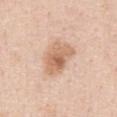<tbp_lesion>
<biopsy_status>not biopsied; imaged during a skin examination</biopsy_status>
<image>
  <source>total-body photography crop</source>
  <field_of_view_mm>15</field_of_view_mm>
</image>
<patient>
  <sex>male</sex>
  <age_approx>50</age_approx>
</patient>
<lighting>white-light</lighting>
<lesion_size>
  <long_diameter_mm_approx>4.5</long_diameter_mm_approx>
</lesion_size>
<site>abdomen</site>
</tbp_lesion>